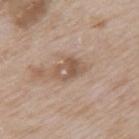The lesion was photographed on a routine skin check and not biopsied; there is no pathology result. On the mid back. A roughly 15 mm field-of-view crop from a total-body skin photograph. The patient is a male in their mid- to late 60s.